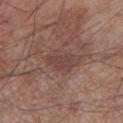Assessment: The lesion was photographed on a routine skin check and not biopsied; there is no pathology result. Image and clinical context: Approximately 3.5 mm at its widest. Cropped from a total-body skin-imaging series; the visible field is about 15 mm. From the left lower leg. The subject is a male aged approximately 55. The lesion-visualizer software estimated a nevus-likeness score of about 0/100 and lesion-presence confidence of about 100/100. Captured under white-light illumination.